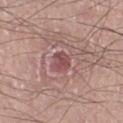Assessment:
This lesion was catalogued during total-body skin photography and was not selected for biopsy.
Image and clinical context:
Located on the left lower leg. The lesion's longest dimension is about 2.5 mm. A male patient, aged 53–57. Cropped from a total-body skin-imaging series; the visible field is about 15 mm. The total-body-photography lesion software estimated a lesion area of about 4.5 mm² and a symmetry-axis asymmetry near 0.2. The software also gave lesion-presence confidence of about 85/100.